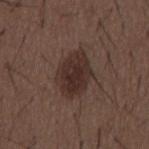  patient:
    sex: male
    age_approx: 50
  image:
    source: total-body photography crop
    field_of_view_mm: 15
  lesion_size:
    long_diameter_mm_approx: 5.0
  lighting: white-light
  site: mid back
  automated_metrics:
    nevus_likeness_0_100: 75
    lesion_detection_confidence_0_100: 100